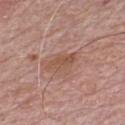biopsy_status: not biopsied; imaged during a skin examination
patient:
  sex: male
  age_approx: 70
site: chest
image:
  source: total-body photography crop
  field_of_view_mm: 15